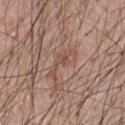Imaged during a routine full-body skin examination; the lesion was not biopsied and no histopathology is available.
Approximately 4.5 mm at its widest.
A 15 mm close-up tile from a total-body photography series done for melanoma screening.
A male patient, aged around 55.
The total-body-photography lesion software estimated an automated nevus-likeness rating near 0 out of 100 and a detector confidence of about 50 out of 100 that the crop contains a lesion.
Located on the chest.
Imaged with white-light lighting.